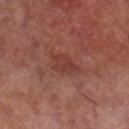biopsy status: imaged on a skin check; not biopsied
TBP lesion metrics: an average lesion color of about L≈37 a*≈25 b*≈25 (CIELAB), a lesion–skin lightness drop of about 6, and a normalized border contrast of about 5.5; a border-irregularity rating of about 3.5/10 and a within-lesion color-variation index near 2.5/10; a classifier nevus-likeness of about 0/100
body site: the right lower leg
lesion size: about 3.5 mm
subject: male, aged 68 to 72
tile lighting: cross-polarized illumination
image: total-body-photography crop, ~15 mm field of view